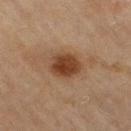{
  "biopsy_status": "not biopsied; imaged during a skin examination",
  "lesion_size": {
    "long_diameter_mm_approx": 4.0
  },
  "image": {
    "source": "total-body photography crop",
    "field_of_view_mm": 15
  },
  "lighting": "cross-polarized",
  "automated_metrics": {
    "cielab_L": 37,
    "cielab_a": 19,
    "cielab_b": 30,
    "vs_skin_darker_L": 12.0,
    "vs_skin_contrast_norm": 10.5,
    "border_irregularity_0_10": 1.5,
    "peripheral_color_asymmetry": 1.0,
    "nevus_likeness_0_100": 100,
    "lesion_detection_confidence_0_100": 100
  },
  "site": "right thigh",
  "patient": {
    "sex": "female",
    "age_approx": 60
  }
}